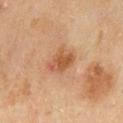  biopsy_status: not biopsied; imaged during a skin examination
  lesion_size:
    long_diameter_mm_approx: 3.5
  site: back
  automated_metrics:
    area_mm2_approx: 8.0
    eccentricity: 0.65
    shape_asymmetry: 0.25
    nevus_likeness_0_100: 55
    lesion_detection_confidence_0_100: 100
  patient:
    sex: male
    age_approx: 45
  image:
    source: total-body photography crop
    field_of_view_mm: 15
  lighting: cross-polarized